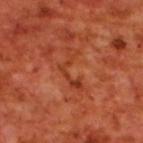- biopsy status · imaged on a skin check; not biopsied
- subject · male, about 70 years old
- lesion diameter · ≈5 mm
- imaging modality · 15 mm crop, total-body photography
- anatomic site · the upper back
- illumination · cross-polarized
- TBP lesion metrics · an area of roughly 5.5 mm², a shape eccentricity near 0.9, and a symmetry-axis asymmetry near 0.85; a lesion-to-skin contrast of about 6 (normalized; higher = more distinct); a within-lesion color-variation index near 0/10 and a peripheral color-asymmetry measure near 0; an automated nevus-likeness rating near 0 out of 100 and lesion-presence confidence of about 90/100